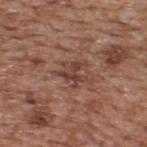Image and clinical context: A roughly 15 mm field-of-view crop from a total-body skin photograph. Imaged with white-light lighting. Longest diameter approximately 4 mm. An algorithmic analysis of the crop reported a lesion area of about 6 mm². And it measured a border-irregularity index near 8.5/10, internal color variation of about 3.5 on a 0–10 scale, and peripheral color asymmetry of about 1. It also reported an automated nevus-likeness rating near 0 out of 100. A male subject roughly 65 years of age. From the upper back.